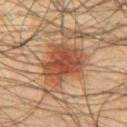notes = no biopsy performed (imaged during a skin exam) | acquisition = 15 mm crop, total-body photography | location = the chest | subject = male, in their 60s | tile lighting = cross-polarized | lesion size = ~5.5 mm (longest diameter) | image-analysis metrics = an automated nevus-likeness rating near 100 out of 100 and a lesion-detection confidence of about 100/100.